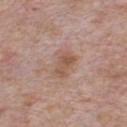Q: Was a biopsy performed?
A: no biopsy performed (imaged during a skin exam)
Q: Lesion location?
A: the chest
Q: Patient demographics?
A: male, aged 58 to 62
Q: What is the imaging modality?
A: ~15 mm crop, total-body skin-cancer survey
Q: Lesion size?
A: ≈3.5 mm